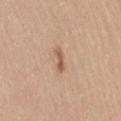Assessment: The lesion was tiled from a total-body skin photograph and was not biopsied. Background: A region of skin cropped from a whole-body photographic capture, roughly 15 mm wide. The patient is a female approximately 45 years of age. The lesion is on the leg.